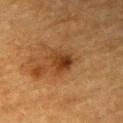Imaged during a routine full-body skin examination; the lesion was not biopsied and no histopathology is available. Approximately 3 mm at its widest. A 15 mm crop from a total-body photograph taken for skin-cancer surveillance. Located on the right upper arm. A male subject, aged 83 or over. The tile uses cross-polarized illumination. An algorithmic analysis of the crop reported a lesion color around L≈35 a*≈21 b*≈34 in CIELAB and a normalized lesion–skin contrast near 8.5. The analysis additionally found a classifier nevus-likeness of about 80/100 and a detector confidence of about 100 out of 100 that the crop contains a lesion.